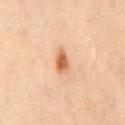The recorded lesion diameter is about 3.5 mm. The lesion is on the mid back. A 15 mm crop from a total-body photograph taken for skin-cancer surveillance. A female patient aged 38 to 42. Imaged with cross-polarized lighting.